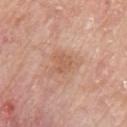Captured during whole-body skin photography for melanoma surveillance; the lesion was not biopsied. This is a white-light tile. The lesion-visualizer software estimated a footprint of about 4 mm², a shape eccentricity near 0.4, and a symmetry-axis asymmetry near 0.3. It also reported a lesion color around L≈58 a*≈22 b*≈32 in CIELAB and roughly 7 lightness units darker than nearby skin. A close-up tile cropped from a whole-body skin photograph, about 15 mm across. The lesion is located on the right upper arm. About 2.5 mm across. The subject is a female aged approximately 65.